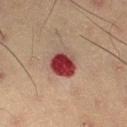Recorded during total-body skin imaging; not selected for excision or biopsy.
Approximately 3.5 mm at its widest.
A 15 mm close-up extracted from a 3D total-body photography capture.
Imaged with cross-polarized lighting.
From the left thigh.
A male subject aged 53 to 57.